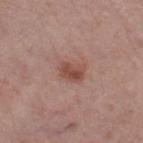* follow-up — no biopsy performed (imaged during a skin exam)
* subject — female, in their mid- to late 60s
* anatomic site — the right thigh
* acquisition — 15 mm crop, total-body photography
* tile lighting — white-light illumination